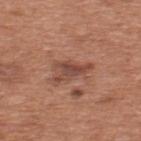biopsy_status: not biopsied; imaged during a skin examination
automated_metrics:
  area_mm2_approx: 6.0
  eccentricity: 0.85
  shape_asymmetry: 0.55
  cielab_L: 46
  cielab_a: 23
  cielab_b: 28
  vs_skin_darker_L: 10.0
lighting: white-light
image:
  source: total-body photography crop
  field_of_view_mm: 15
patient:
  sex: male
  age_approx: 65
lesion_size:
  long_diameter_mm_approx: 4.0
site: upper back